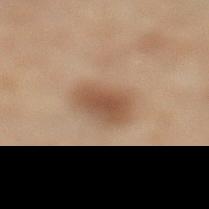The lesion was photographed on a routine skin check and not biopsied; there is no pathology result.
Measured at roughly 4 mm in maximum diameter.
A close-up tile cropped from a whole-body skin photograph, about 15 mm across.
A male subject, aged approximately 70.
Automated tile analysis of the lesion measured a footprint of about 9.5 mm², a shape eccentricity near 0.75, and two-axis asymmetry of about 0.2. The analysis additionally found a nevus-likeness score of about 85/100 and a lesion-detection confidence of about 100/100.
The tile uses cross-polarized illumination.
On the leg.